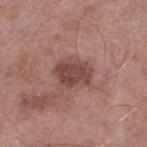  biopsy_status: not biopsied; imaged during a skin examination
  lighting: white-light
  lesion_size:
    long_diameter_mm_approx: 4.0
  patient:
    sex: male
    age_approx: 55
  site: left lower leg
  image:
    source: total-body photography crop
    field_of_view_mm: 15